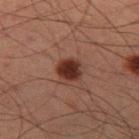Clinical impression: Captured during whole-body skin photography for melanoma surveillance; the lesion was not biopsied. Background: The lesion is on the left thigh. A close-up tile cropped from a whole-body skin photograph, about 15 mm across. A male subject, aged around 60.